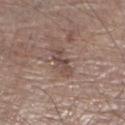Assessment: No biopsy was performed on this lesion — it was imaged during a full skin examination and was not determined to be concerning. Clinical summary: Located on the right lower leg. About 4 mm across. Captured under white-light illumination. A male subject, about 80 years old. The lesion-visualizer software estimated a lesion color around L≈47 a*≈16 b*≈21 in CIELAB, roughly 9 lightness units darker than nearby skin, and a normalized lesion–skin contrast near 7. It also reported a detector confidence of about 65 out of 100 that the crop contains a lesion. A 15 mm close-up extracted from a 3D total-body photography capture.